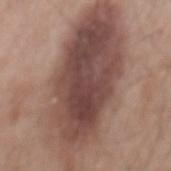The lesion was photographed on a routine skin check and not biopsied; there is no pathology result. The lesion is on the mid back. The lesion-visualizer software estimated a lesion area of about 75 mm². It also reported a mean CIELAB color near L≈47 a*≈18 b*≈22, roughly 14 lightness units darker than nearby skin, and a lesion-to-skin contrast of about 10 (normalized; higher = more distinct). The analysis additionally found a nevus-likeness score of about 80/100 and a lesion-detection confidence of about 100/100. A lesion tile, about 15 mm wide, cut from a 3D total-body photograph. A male subject, aged around 55. The lesion's longest dimension is about 16.5 mm.